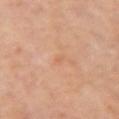| key | value |
|---|---|
| workup | imaged on a skin check; not biopsied |
| image | total-body-photography crop, ~15 mm field of view |
| site | the right upper arm |
| lesion diameter | ~1 mm (longest diameter) |
| subject | male, aged 68 to 72 |